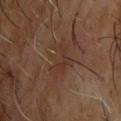workup: catalogued during a skin exam; not biopsied
lesion diameter: ~4 mm (longest diameter)
anatomic site: the upper back
imaging modality: ~15 mm tile from a whole-body skin photo
lighting: cross-polarized illumination
subject: male, aged 63–67